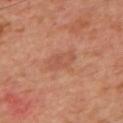follow-up — imaged on a skin check; not biopsied
lighting — cross-polarized
anatomic site — the upper back
image — ~15 mm tile from a whole-body skin photo
automated lesion analysis — a lesion area of about 6.5 mm², an eccentricity of roughly 0.85, and two-axis asymmetry of about 0.25; peripheral color asymmetry of about 1
subject — male, aged 58 to 62
size — ~4 mm (longest diameter)